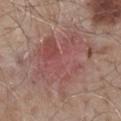Imaged during a routine full-body skin examination; the lesion was not biopsied and no histopathology is available. Imaged with white-light lighting. A male subject in their mid- to late 50s. Located on the back. The recorded lesion diameter is about 11.5 mm. A 15 mm crop from a total-body photograph taken for skin-cancer surveillance.